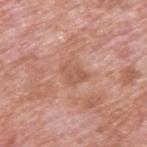| field | value |
|---|---|
| biopsy status | total-body-photography surveillance lesion; no biopsy |
| lesion diameter | about 3 mm |
| image source | ~15 mm crop, total-body skin-cancer survey |
| subject | male, aged 58 to 62 |
| site | the upper back |
| tile lighting | white-light |